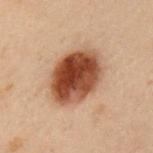biopsy_status: not biopsied; imaged during a skin examination
site: left upper arm
lighting: cross-polarized
automated_metrics:
  cielab_L: 38
  cielab_a: 21
  cielab_b: 28
  vs_skin_darker_L: 17.0
  border_irregularity_0_10: 1.0
  color_variation_0_10: 7.5
  peripheral_color_asymmetry: 2.5
patient:
  sex: male
  age_approx: 55
image:
  source: total-body photography crop
  field_of_view_mm: 15